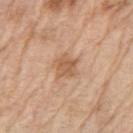This lesion was catalogued during total-body skin photography and was not selected for biopsy.
The lesion is located on the left upper arm.
Cropped from a whole-body photographic skin survey; the tile spans about 15 mm.
The patient is a male aged 68–72.
About 3.5 mm across.
An algorithmic analysis of the crop reported a border-irregularity rating of about 3/10 and a color-variation rating of about 2/10.
Imaged with white-light lighting.